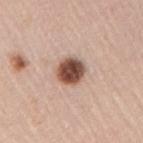Captured during whole-body skin photography for melanoma surveillance; the lesion was not biopsied. The tile uses white-light illumination. The subject is a male about 45 years old. A roughly 15 mm field-of-view crop from a total-body skin photograph. From the right upper arm. The lesion's longest dimension is about 3 mm.